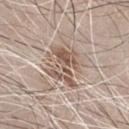{
  "biopsy_status": "not biopsied; imaged during a skin examination",
  "site": "chest",
  "image": {
    "source": "total-body photography crop",
    "field_of_view_mm": 15
  },
  "patient": {
    "sex": "male",
    "age_approx": 65
  },
  "lesion_size": {
    "long_diameter_mm_approx": 5.0
  }
}